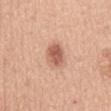• biopsy status — no biopsy performed (imaged during a skin exam)
• acquisition — ~15 mm tile from a whole-body skin photo
• illumination — white-light illumination
• site — the mid back
• subject — male, in their 50s